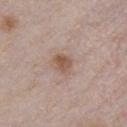| feature | finding |
|---|---|
| biopsy status | total-body-photography surveillance lesion; no biopsy |
| tile lighting | white-light illumination |
| image source | total-body-photography crop, ~15 mm field of view |
| subject | male, aged 28 to 32 |
| automated lesion analysis | an area of roughly 4 mm², an eccentricity of roughly 0.55, and a shape-asymmetry score of about 0.25 (0 = symmetric) |
| size | about 2.5 mm |